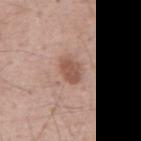Recorded during total-body skin imaging; not selected for excision or biopsy. About 3 mm across. The total-body-photography lesion software estimated a lesion area of about 5.5 mm² and an outline eccentricity of about 0.65 (0 = round, 1 = elongated). The analysis additionally found border irregularity of about 1.5 on a 0–10 scale, a within-lesion color-variation index near 1.5/10, and peripheral color asymmetry of about 0.5. The analysis additionally found a classifier nevus-likeness of about 85/100. Imaged with white-light lighting. A roughly 15 mm field-of-view crop from a total-body skin photograph. From the mid back. A male subject, aged around 55.